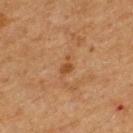follow-up: imaged on a skin check; not biopsied | automated lesion analysis: border irregularity of about 4.5 on a 0–10 scale and a within-lesion color-variation index near 0.5/10 | size: about 2.5 mm | subject: male, roughly 50 years of age | lighting: cross-polarized | image source: ~15 mm tile from a whole-body skin photo | location: the upper back.